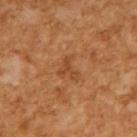The lesion was tiled from a total-body skin photograph and was not biopsied. A male patient, aged around 65. The total-body-photography lesion software estimated a border-irregularity index near 7.5/10, a within-lesion color-variation index near 0/10, and a peripheral color-asymmetry measure near 0. And it measured a classifier nevus-likeness of about 0/100. The tile uses cross-polarized illumination. Measured at roughly 2.5 mm in maximum diameter. A region of skin cropped from a whole-body photographic capture, roughly 15 mm wide.